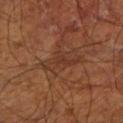| field | value |
|---|---|
| follow-up | catalogued during a skin exam; not biopsied |
| location | the left lower leg |
| image source | ~15 mm crop, total-body skin-cancer survey |
| patient | approximately 65 years of age |
| automated metrics | a footprint of about 5.5 mm², an outline eccentricity of about 0.9 (0 = round, 1 = elongated), and a symmetry-axis asymmetry near 0.65; a lesion color around L≈34 a*≈22 b*≈28 in CIELAB and about 6 CIELAB-L* units darker than the surrounding skin; a classifier nevus-likeness of about 0/100 |
| illumination | cross-polarized |
| lesion diameter | about 5 mm |